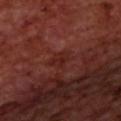Acquisition and patient details:
The lesion is on the upper back. A region of skin cropped from a whole-body photographic capture, roughly 15 mm wide. A male subject approximately 70 years of age.